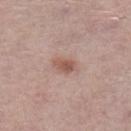Assessment: Part of a total-body skin-imaging series; this lesion was reviewed on a skin check and was not flagged for biopsy. Background: The tile uses white-light illumination. The lesion's longest dimension is about 3 mm. Located on the left lower leg. A female subject, in their mid- to late 60s. A region of skin cropped from a whole-body photographic capture, roughly 15 mm wide.